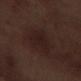<record>
  <biopsy_status>not biopsied; imaged during a skin examination</biopsy_status>
  <patient>
    <sex>male</sex>
    <age_approx>70</age_approx>
  </patient>
  <lighting>white-light</lighting>
  <image>
    <source>total-body photography crop</source>
    <field_of_view_mm>15</field_of_view_mm>
  </image>
  <lesion_size>
    <long_diameter_mm_approx>4.5</long_diameter_mm_approx>
  </lesion_size>
  <site>right lower leg</site>
</record>